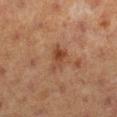Background:
A lesion tile, about 15 mm wide, cut from a 3D total-body photograph. Imaged with cross-polarized lighting. A female patient approximately 40 years of age. Longest diameter approximately 3.5 mm. Automated image analysis of the tile measured an area of roughly 5.5 mm², an outline eccentricity of about 0.8 (0 = round, 1 = elongated), and two-axis asymmetry of about 0.4. And it measured border irregularity of about 4 on a 0–10 scale, internal color variation of about 5 on a 0–10 scale, and radial color variation of about 2. The lesion is located on the right lower leg.